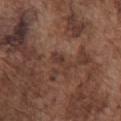{
  "biopsy_status": "not biopsied; imaged during a skin examination",
  "lesion_size": {
    "long_diameter_mm_approx": 3.0
  },
  "automated_metrics": {
    "nevus_likeness_0_100": 0,
    "lesion_detection_confidence_0_100": 95
  },
  "lighting": "white-light",
  "image": {
    "source": "total-body photography crop",
    "field_of_view_mm": 15
  },
  "site": "chest",
  "patient": {
    "sex": "male",
    "age_approx": 75
  }
}